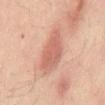| key | value |
|---|---|
| biopsy status | no biopsy performed (imaged during a skin exam) |
| size | ~6.5 mm (longest diameter) |
| body site | the mid back |
| image-analysis metrics | a symmetry-axis asymmetry near 0.2; roughly 10 lightness units darker than nearby skin; a border-irregularity rating of about 3/10 and internal color variation of about 2.5 on a 0–10 scale |
| imaging modality | ~15 mm tile from a whole-body skin photo |
| subject | male, aged 48–52 |